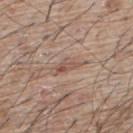Recorded during total-body skin imaging; not selected for excision or biopsy. The tile uses white-light illumination. A male subject, approximately 65 years of age. The lesion is on the upper back. This image is a 15 mm lesion crop taken from a total-body photograph. The lesion's longest dimension is about 3 mm.